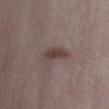anatomic site = the left lower leg; tile lighting = white-light illumination; acquisition = total-body-photography crop, ~15 mm field of view; patient = female, aged 43–47; size = about 4 mm.